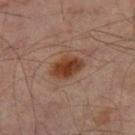follow-up: no biopsy performed (imaged during a skin exam); image: 15 mm crop, total-body photography; location: the leg; patient: male, aged around 65.